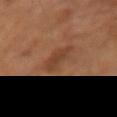No biopsy was performed on this lesion — it was imaged during a full skin examination and was not determined to be concerning.
Located on the right forearm.
The tile uses cross-polarized illumination.
The patient is a female in their 60s.
Cropped from a whole-body photographic skin survey; the tile spans about 15 mm.